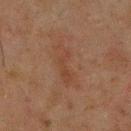Part of a total-body skin-imaging series; this lesion was reviewed on a skin check and was not flagged for biopsy. This image is a 15 mm lesion crop taken from a total-body photograph. A male subject in their mid-40s. The lesion is on the back. About 6 mm across. An algorithmic analysis of the crop reported border irregularity of about 6 on a 0–10 scale, a color-variation rating of about 2.5/10, and radial color variation of about 1.